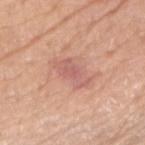workup — total-body-photography surveillance lesion; no biopsy
patient — male, aged 78–82
illumination — white-light illumination
location — the right upper arm
acquisition — 15 mm crop, total-body photography